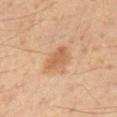Clinical impression: This lesion was catalogued during total-body skin photography and was not selected for biopsy. Image and clinical context: A 15 mm close-up tile from a total-body photography series done for melanoma screening. A male patient aged 48 to 52. Imaged with cross-polarized lighting. About 2.5 mm across. The lesion is located on the left lower leg.